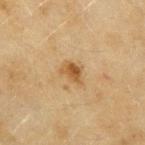• workup: total-body-photography surveillance lesion; no biopsy
• patient: female, aged 53 to 57
• image: ~15 mm crop, total-body skin-cancer survey
• illumination: cross-polarized illumination
• anatomic site: the left upper arm